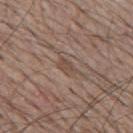Captured during whole-body skin photography for melanoma surveillance; the lesion was not biopsied. The subject is a male aged 63 to 67. On the mid back. The tile uses white-light illumination. Approximately 2.5 mm at its widest. A lesion tile, about 15 mm wide, cut from a 3D total-body photograph.